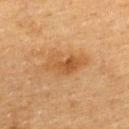{
  "image": {
    "source": "total-body photography crop",
    "field_of_view_mm": 15
  },
  "lesion_size": {
    "long_diameter_mm_approx": 5.0
  },
  "patient": {
    "sex": "female",
    "age_approx": 55
  },
  "site": "upper back"
}